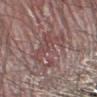biopsy_status: not biopsied; imaged during a skin examination
automated_metrics:
  area_mm2_approx: 15.0
  eccentricity: 0.8
  shape_asymmetry: 0.45
  cielab_L: 47
  cielab_a: 20
  cielab_b: 19
  border_irregularity_0_10: 8.5
lesion_size:
  long_diameter_mm_approx: 7.0
patient:
  sex: male
  age_approx: 50
site: leg
image:
  source: total-body photography crop
  field_of_view_mm: 15
lighting: white-light